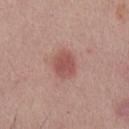Findings:
- follow-up — no biopsy performed (imaged during a skin exam)
- patient — male, aged approximately 25
- image — ~15 mm crop, total-body skin-cancer survey
- anatomic site — the front of the torso